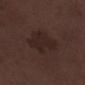Q: Was a biopsy performed?
A: catalogued during a skin exam; not biopsied
Q: Illumination type?
A: white-light
Q: How large is the lesion?
A: ≈5.5 mm
Q: Lesion location?
A: the right lower leg
Q: Patient demographics?
A: male, aged around 70
Q: How was this image acquired?
A: ~15 mm tile from a whole-body skin photo